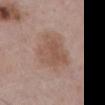Background: Located on the abdomen. A 15 mm crop from a total-body photograph taken for skin-cancer surveillance. Approximately 5 mm at its widest. A male subject approximately 75 years of age. The lesion-visualizer software estimated a mean CIELAB color near L≈53 a*≈19 b*≈26 and roughly 8 lightness units darker than nearby skin. And it measured a border-irregularity rating of about 3/10, a color-variation rating of about 3/10, and radial color variation of about 1.